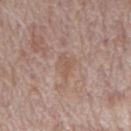The subject is a male about 70 years old. The lesion is located on the back. Approximately 2.5 mm at its widest. A 15 mm crop from a total-body photograph taken for skin-cancer surveillance. Imaged with white-light lighting. Automated image analysis of the tile measured a border-irregularity rating of about 6/10 and internal color variation of about 0 on a 0–10 scale.